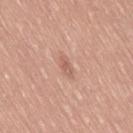Captured during whole-body skin photography for melanoma surveillance; the lesion was not biopsied. The total-body-photography lesion software estimated an area of roughly 3 mm², an outline eccentricity of about 0.9 (0 = round, 1 = elongated), and two-axis asymmetry of about 0.25. The software also gave a border-irregularity rating of about 3/10, a within-lesion color-variation index near 0/10, and peripheral color asymmetry of about 0. A male subject, approximately 60 years of age. A close-up tile cropped from a whole-body skin photograph, about 15 mm across. The lesion is on the lower back.